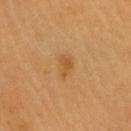Findings:
- notes: catalogued during a skin exam; not biopsied
- diameter: about 3 mm
- image source: total-body-photography crop, ~15 mm field of view
- automated lesion analysis: about 7 CIELAB-L* units darker than the surrounding skin and a lesion-to-skin contrast of about 5.5 (normalized; higher = more distinct)
- patient: male, about 60 years old
- site: the head or neck
- illumination: cross-polarized illumination